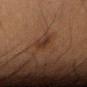Case summary:
* workup · catalogued during a skin exam; not biopsied
* body site · the lower back
* image source · 15 mm crop, total-body photography
* lesion diameter · ~2.5 mm (longest diameter)
* patient · male, aged approximately 55
* automated metrics · an outline eccentricity of about 0.5 (0 = round, 1 = elongated) and a shape-asymmetry score of about 0.4 (0 = symmetric); a border-irregularity rating of about 3.5/10 and a within-lesion color-variation index near 2/10; a nevus-likeness score of about 45/100